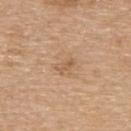Clinical impression: Recorded during total-body skin imaging; not selected for excision or biopsy. Image and clinical context: A male subject, about 70 years old. Automated tile analysis of the lesion measured an average lesion color of about L≈58 a*≈18 b*≈34 (CIELAB), about 8 CIELAB-L* units darker than the surrounding skin, and a normalized lesion–skin contrast near 5. Imaged with white-light lighting. The lesion is located on the upper back. A 15 mm crop from a total-body photograph taken for skin-cancer surveillance.